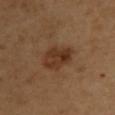{"biopsy_status": "not biopsied; imaged during a skin examination", "patient": {"sex": "female", "age_approx": 40}, "image": {"source": "total-body photography crop", "field_of_view_mm": 15}, "site": "upper back"}